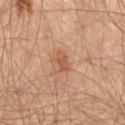workup: imaged on a skin check; not biopsied | lighting: cross-polarized | lesion size: ≈2.5 mm | site: the left thigh | patient: male, in their mid- to late 60s | image: 15 mm crop, total-body photography.